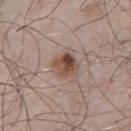  biopsy_status: not biopsied; imaged during a skin examination
  automated_metrics:
    border_irregularity_0_10: 1.5
    nevus_likeness_0_100: 95
    lesion_detection_confidence_0_100: 100
  lighting: white-light
  lesion_size:
    long_diameter_mm_approx: 3.0
  image:
    source: total-body photography crop
    field_of_view_mm: 15
  patient:
    sex: male
    age_approx: 55
  site: front of the torso